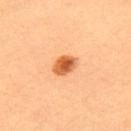Notes:
– patient — female, approximately 35 years of age
– image — 15 mm crop, total-body photography
– tile lighting — cross-polarized
– site — the upper back
– size — ~3 mm (longest diameter)
– automated lesion analysis — an average lesion color of about L≈60 a*≈30 b*≈45 (CIELAB), about 16 CIELAB-L* units darker than the surrounding skin, and a lesion-to-skin contrast of about 10 (normalized; higher = more distinct); border irregularity of about 2 on a 0–10 scale, a within-lesion color-variation index near 6/10, and peripheral color asymmetry of about 2; an automated nevus-likeness rating near 100 out of 100 and a lesion-detection confidence of about 100/100